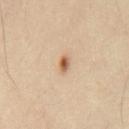Cropped from a total-body skin-imaging series; the visible field is about 15 mm. Approximately 2 mm at its widest. An algorithmic analysis of the crop reported a footprint of about 2.5 mm², an outline eccentricity of about 0.8 (0 = round, 1 = elongated), and a symmetry-axis asymmetry near 0.25. The software also gave an average lesion color of about L≈59 a*≈20 b*≈35 (CIELAB) and about 14 CIELAB-L* units darker than the surrounding skin. A male patient about 40 years old. The tile uses cross-polarized illumination. From the chest.